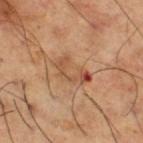{
  "patient": {
    "sex": "male",
    "age_approx": 65
  },
  "site": "right thigh",
  "image": {
    "source": "total-body photography crop",
    "field_of_view_mm": 15
  },
  "automated_metrics": {
    "area_mm2_approx": 6.0,
    "eccentricity": 0.9,
    "shape_asymmetry": 0.55,
    "nevus_likeness_0_100": 0
  },
  "lesion_size": {
    "long_diameter_mm_approx": 4.0
  },
  "lighting": "cross-polarized"
}